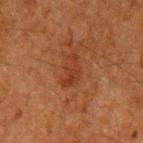Clinical impression: Part of a total-body skin-imaging series; this lesion was reviewed on a skin check and was not flagged for biopsy. Background: Captured under cross-polarized illumination. A roughly 15 mm field-of-view crop from a total-body skin photograph. Automated image analysis of the tile measured a within-lesion color-variation index near 2/10 and radial color variation of about 0.5. From the left upper arm. The recorded lesion diameter is about 4 mm. The patient is a male aged 58 to 62.